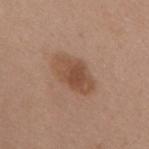patient: female, aged around 65 | imaging modality: ~15 mm crop, total-body skin-cancer survey | lesion size: ≈5.5 mm | TBP lesion metrics: about 10 CIELAB-L* units darker than the surrounding skin and a lesion-to-skin contrast of about 7.5 (normalized; higher = more distinct); a border-irregularity rating of about 2/10 and a peripheral color-asymmetry measure near 1; an automated nevus-likeness rating near 80 out of 100 | site: the back | tile lighting: white-light illumination.